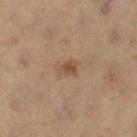| field | value |
|---|---|
| biopsy status | total-body-photography surveillance lesion; no biopsy |
| lesion diameter | ~2 mm (longest diameter) |
| subject | female, aged 38–42 |
| image source | 15 mm crop, total-body photography |
| TBP lesion metrics | an area of roughly 3 mm², an outline eccentricity of about 0.65 (0 = round, 1 = elongated), and a shape-asymmetry score of about 0.35 (0 = symmetric) |
| lighting | cross-polarized |
| site | the right thigh |